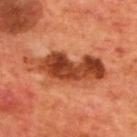workup: imaged on a skin check; not biopsied | tile lighting: cross-polarized | lesion diameter: about 9.5 mm | acquisition: 15 mm crop, total-body photography | automated metrics: an area of roughly 22 mm² and an outline eccentricity of about 0.95 (0 = round, 1 = elongated); a border-irregularity rating of about 5.5/10, a color-variation rating of about 6.5/10, and a peripheral color-asymmetry measure near 2.5; a detector confidence of about 100 out of 100 that the crop contains a lesion | subject: male, about 70 years old | location: the mid back.